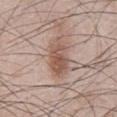{
  "biopsy_status": "not biopsied; imaged during a skin examination"
}